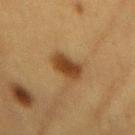follow-up: catalogued during a skin exam; not biopsied
lighting: cross-polarized illumination
patient: female, in their mid-50s
image-analysis metrics: an eccentricity of roughly 0.65; peripheral color asymmetry of about 1
image source: 15 mm crop, total-body photography
anatomic site: the mid back